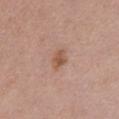Impression:
The lesion was tiled from a total-body skin photograph and was not biopsied.
Context:
A 15 mm crop from a total-body photograph taken for skin-cancer surveillance. The subject is a female aged 43 to 47. The tile uses white-light illumination. An algorithmic analysis of the crop reported about 9 CIELAB-L* units darker than the surrounding skin. The software also gave a border-irregularity rating of about 2/10. The lesion is located on the chest. Measured at roughly 2.5 mm in maximum diameter.